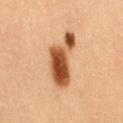biopsy_status: not biopsied; imaged during a skin examination
lighting: cross-polarized
automated_metrics:
  eccentricity: 0.9
  shape_asymmetry: 0.5
  cielab_L: 48
  cielab_a: 24
  cielab_b: 36
  vs_skin_darker_L: 18.0
  vs_skin_contrast_norm: 12.5
  lesion_detection_confidence_0_100: 100
lesion_size:
  long_diameter_mm_approx: 6.5
site: back
image:
  source: total-body photography crop
  field_of_view_mm: 15
patient:
  sex: female
  age_approx: 30